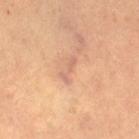Clinical impression:
Recorded during total-body skin imaging; not selected for excision or biopsy.
Acquisition and patient details:
Captured under cross-polarized illumination. This image is a 15 mm lesion crop taken from a total-body photograph. Approximately 3 mm at its widest. A female subject, aged 28–32. Automated image analysis of the tile measured a lesion area of about 2.5 mm², an outline eccentricity of about 0.95 (0 = round, 1 = elongated), and a shape-asymmetry score of about 0.45 (0 = symmetric). The analysis additionally found an average lesion color of about L≈61 a*≈21 b*≈29 (CIELAB) and a lesion–skin lightness drop of about 7. The software also gave a border-irregularity rating of about 6.5/10, internal color variation of about 0 on a 0–10 scale, and radial color variation of about 0. It also reported an automated nevus-likeness rating near 0 out of 100 and a lesion-detection confidence of about 90/100. On the leg.